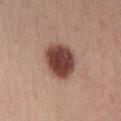<case>
<biopsy_status>not biopsied; imaged during a skin examination</biopsy_status>
<site>chest</site>
<image>
  <source>total-body photography crop</source>
  <field_of_view_mm>15</field_of_view_mm>
</image>
<patient>
  <sex>female</sex>
  <age_approx>25</age_approx>
</patient>
<lesion_size>
  <long_diameter_mm_approx>5.0</long_diameter_mm_approx>
</lesion_size>
</case>